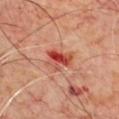No biopsy was performed on this lesion — it was imaged during a full skin examination and was not determined to be concerning.
A male subject, roughly 70 years of age.
From the chest.
Cropped from a total-body skin-imaging series; the visible field is about 15 mm.